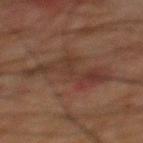This lesion was catalogued during total-body skin photography and was not selected for biopsy. A 15 mm crop from a total-body photograph taken for skin-cancer surveillance. From the mid back. A male patient, about 65 years old. Automated tile analysis of the lesion measured a classifier nevus-likeness of about 0/100 and a lesion-detection confidence of about 80/100. The recorded lesion diameter is about 8.5 mm. Captured under cross-polarized illumination.